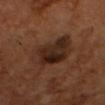subject: male, aged 48 to 52 | automated lesion analysis: an outline eccentricity of about 0.6 (0 = round, 1 = elongated); border irregularity of about 2.5 on a 0–10 scale, a color-variation rating of about 7.5/10, and peripheral color asymmetry of about 2.5; an automated nevus-likeness rating near 95 out of 100 | lighting: cross-polarized illumination | image source: 15 mm crop, total-body photography | lesion diameter: ~5.5 mm (longest diameter) | location: the head or neck.